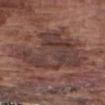Captured during whole-body skin photography for melanoma surveillance; the lesion was not biopsied. A male subject about 75 years old. On the arm. A 15 mm close-up tile from a total-body photography series done for melanoma screening.